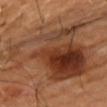Q: Was this lesion biopsied?
A: catalogued during a skin exam; not biopsied
Q: Patient demographics?
A: male, aged approximately 65
Q: Lesion location?
A: the chest
Q: Lesion size?
A: about 14 mm
Q: How was this image acquired?
A: ~15 mm crop, total-body skin-cancer survey
Q: What lighting was used for the tile?
A: cross-polarized illumination